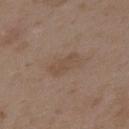image:
  source: total-body photography crop
  field_of_view_mm: 15
patient:
  sex: female
  age_approx: 35
automated_metrics:
  area_mm2_approx: 6.0
  shape_asymmetry: 0.2
  cielab_L: 48
  cielab_a: 15
  cielab_b: 27
  vs_skin_darker_L: 6.0
  vs_skin_contrast_norm: 5.0
  border_irregularity_0_10: 2.0
  color_variation_0_10: 1.5
  peripheral_color_asymmetry: 0.5
site: upper back